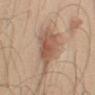Q: Was this lesion biopsied?
A: total-body-photography surveillance lesion; no biopsy
Q: Lesion size?
A: ~5.5 mm (longest diameter)
Q: What is the imaging modality?
A: ~15 mm crop, total-body skin-cancer survey
Q: Who is the patient?
A: male, in their mid-40s
Q: How was the tile lit?
A: white-light
Q: What is the anatomic site?
A: the right upper arm